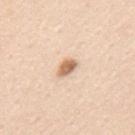Q: Was this lesion biopsied?
A: catalogued during a skin exam; not biopsied
Q: What kind of image is this?
A: ~15 mm crop, total-body skin-cancer survey
Q: What are the patient's age and sex?
A: female, aged 43–47
Q: What did automated image analysis measure?
A: a lesion color around L≈67 a*≈19 b*≈33 in CIELAB, about 15 CIELAB-L* units darker than the surrounding skin, and a lesion-to-skin contrast of about 9 (normalized; higher = more distinct); a border-irregularity rating of about 1.5/10, a within-lesion color-variation index near 3/10, and radial color variation of about 1
Q: Lesion size?
A: ≈2.5 mm
Q: What is the anatomic site?
A: the mid back
Q: Illumination type?
A: white-light illumination